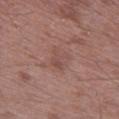Clinical impression: This lesion was catalogued during total-body skin photography and was not selected for biopsy. Clinical summary: The lesion's longest dimension is about 3 mm. A male subject aged 48–52. The lesion-visualizer software estimated a lesion–skin lightness drop of about 6 and a normalized border contrast of about 4.5. The analysis additionally found a border-irregularity index near 5.5/10 and radial color variation of about 0.5. And it measured a classifier nevus-likeness of about 0/100 and lesion-presence confidence of about 100/100. A close-up tile cropped from a whole-body skin photograph, about 15 mm across. This is a white-light tile. The lesion is located on the left thigh.